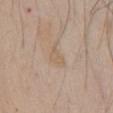The lesion was photographed on a routine skin check and not biopsied; there is no pathology result.
A 15 mm crop from a total-body photograph taken for skin-cancer surveillance.
The subject is a male aged 43 to 47.
From the chest.